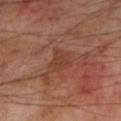{"biopsy_status": "not biopsied; imaged during a skin examination", "patient": {"sex": "male", "age_approx": 70}, "image": {"source": "total-body photography crop", "field_of_view_mm": 15}, "lesion_size": {"long_diameter_mm_approx": 3.0}, "site": "left lower leg", "lighting": "cross-polarized"}